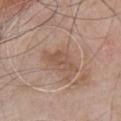Case summary:
* workup — catalogued during a skin exam; not biopsied
* site — the chest
* patient — male, in their mid-50s
* lesion size — ≈4 mm
* tile lighting — white-light
* acquisition — total-body-photography crop, ~15 mm field of view
* TBP lesion metrics — a border-irregularity rating of about 5.5/10, a within-lesion color-variation index near 1/10, and a peripheral color-asymmetry measure near 0.5